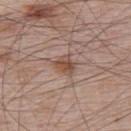Assessment: The lesion was tiled from a total-body skin photograph and was not biopsied. Background: Longest diameter approximately 2.5 mm. From the back. A male subject aged 63–67. Imaged with white-light lighting. Cropped from a total-body skin-imaging series; the visible field is about 15 mm.